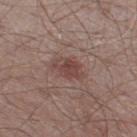Q: Is there a histopathology result?
A: imaged on a skin check; not biopsied
Q: What is the anatomic site?
A: the right thigh
Q: How was the tile lit?
A: white-light
Q: What is the lesion's diameter?
A: about 4 mm
Q: What is the imaging modality?
A: ~15 mm crop, total-body skin-cancer survey
Q: What are the patient's age and sex?
A: male, aged 53 to 57
Q: Automated lesion metrics?
A: a lesion color around L≈44 a*≈20 b*≈21 in CIELAB, a lesion–skin lightness drop of about 8, and a lesion-to-skin contrast of about 6.5 (normalized; higher = more distinct); a border-irregularity index near 3/10 and internal color variation of about 3.5 on a 0–10 scale; a lesion-detection confidence of about 100/100